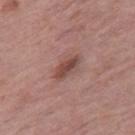notes = no biopsy performed (imaged during a skin exam)
size = ~4 mm (longest diameter)
site = the right thigh
image = 15 mm crop, total-body photography
subject = female, approximately 65 years of age
illumination = white-light
TBP lesion metrics = an average lesion color of about L≈47 a*≈21 b*≈23 (CIELAB), a lesion–skin lightness drop of about 10, and a normalized lesion–skin contrast near 7.5